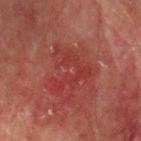Clinical impression:
The lesion was tiled from a total-body skin photograph and was not biopsied.
Clinical summary:
The lesion is located on the left upper arm. Captured under cross-polarized illumination. About 5 mm across. A male subject in their mid- to late 70s. The lesion-visualizer software estimated an area of roughly 9.5 mm² and an eccentricity of roughly 0.6. The analysis additionally found a lesion color around L≈32 a*≈30 b*≈23 in CIELAB. And it measured a border-irregularity rating of about 10/10 and radial color variation of about 0.5. It also reported an automated nevus-likeness rating near 0 out of 100 and lesion-presence confidence of about 90/100. A region of skin cropped from a whole-body photographic capture, roughly 15 mm wide.